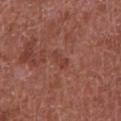Recorded during total-body skin imaging; not selected for excision or biopsy. Located on the abdomen. Automated tile analysis of the lesion measured a shape eccentricity near 0.9. It also reported a mean CIELAB color near L≈41 a*≈26 b*≈28, roughly 6 lightness units darker than nearby skin, and a lesion-to-skin contrast of about 5.5 (normalized; higher = more distinct). A 15 mm close-up tile from a total-body photography series done for melanoma screening. A male patient aged 63 to 67. Longest diameter approximately 3 mm. The tile uses white-light illumination.